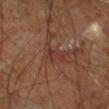Captured during whole-body skin photography for melanoma surveillance; the lesion was not biopsied. The lesion is on the right leg. A region of skin cropped from a whole-body photographic capture, roughly 15 mm wide. The tile uses cross-polarized illumination. Measured at roughly 2.5 mm in maximum diameter. A male subject, aged 58–62. The total-body-photography lesion software estimated a shape eccentricity near 0.85 and a shape-asymmetry score of about 0.4 (0 = symmetric). The software also gave a detector confidence of about 70 out of 100 that the crop contains a lesion.